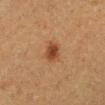Case summary:
– biopsy status · no biopsy performed (imaged during a skin exam)
– subject · female, aged around 40
– lighting · cross-polarized
– diameter · about 2.5 mm
– image · ~15 mm crop, total-body skin-cancer survey
– site · the left lower leg
– automated metrics · a within-lesion color-variation index near 2.5/10 and peripheral color asymmetry of about 1